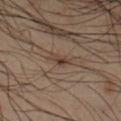Q: What did automated image analysis measure?
A: an area of roughly 3 mm² and a shape-asymmetry score of about 0.35 (0 = symmetric); a border-irregularity rating of about 3.5/10, internal color variation of about 3 on a 0–10 scale, and radial color variation of about 1; a nevus-likeness score of about 45/100 and a lesion-detection confidence of about 100/100
Q: What are the patient's age and sex?
A: male, about 40 years old
Q: What lighting was used for the tile?
A: cross-polarized
Q: What is the imaging modality?
A: ~15 mm crop, total-body skin-cancer survey
Q: Lesion size?
A: ≈2.5 mm
Q: Lesion location?
A: the arm